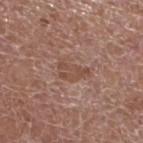anatomic site: the left lower leg; lesion size: ≈4 mm; image-analysis metrics: a nevus-likeness score of about 0/100 and lesion-presence confidence of about 100/100; acquisition: ~15 mm crop, total-body skin-cancer survey; subject: male, aged approximately 75; lighting: white-light.